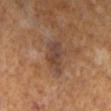• biopsy status — catalogued during a skin exam; not biopsied
• lesion size — ≈4.5 mm
• image-analysis metrics — a lesion color around L≈40 a*≈17 b*≈25 in CIELAB, about 8 CIELAB-L* units darker than the surrounding skin, and a lesion-to-skin contrast of about 8 (normalized; higher = more distinct); a nevus-likeness score of about 0/100
• illumination — cross-polarized
• imaging modality — total-body-photography crop, ~15 mm field of view
• patient — female, aged around 65
• body site — the left lower leg